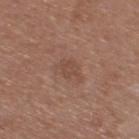Q: Was a biopsy performed?
A: catalogued during a skin exam; not biopsied
Q: How large is the lesion?
A: ≈3.5 mm
Q: What kind of image is this?
A: total-body-photography crop, ~15 mm field of view
Q: What are the patient's age and sex?
A: male, aged 73 to 77
Q: What is the anatomic site?
A: the right thigh
Q: Illumination type?
A: white-light illumination